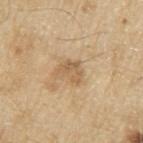biopsy_status: not biopsied; imaged during a skin examination